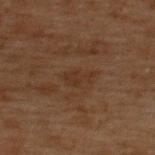Part of a total-body skin-imaging series; this lesion was reviewed on a skin check and was not flagged for biopsy. A close-up tile cropped from a whole-body skin photograph, about 15 mm across. A male subject approximately 70 years of age. The lesion's longest dimension is about 3 mm. Automated image analysis of the tile measured a border-irregularity index near 5/10, a color-variation rating of about 1/10, and radial color variation of about 0.5. Located on the upper back. The tile uses cross-polarized illumination.